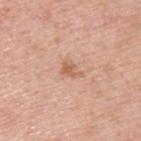The lesion was photographed on a routine skin check and not biopsied; there is no pathology result.
The lesion is located on the upper back.
A male subject, aged 48–52.
Measured at roughly 2.5 mm in maximum diameter.
Cropped from a total-body skin-imaging series; the visible field is about 15 mm.
The tile uses white-light illumination.